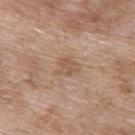notes: total-body-photography surveillance lesion; no biopsy | body site: the upper back | imaging modality: 15 mm crop, total-body photography | subject: female, aged 73 to 77.